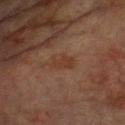Acquisition and patient details: On the front of the torso. Cropped from a whole-body photographic skin survey; the tile spans about 15 mm. The patient is a male aged approximately 75.